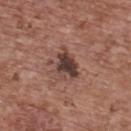This lesion was catalogued during total-body skin photography and was not selected for biopsy. The recorded lesion diameter is about 4 mm. A close-up tile cropped from a whole-body skin photograph, about 15 mm across. A female subject, aged 63 to 67. Captured under white-light illumination. The lesion is located on the upper back. Automated tile analysis of the lesion measured a lesion area of about 9 mm² and an outline eccentricity of about 0.55 (0 = round, 1 = elongated). The analysis additionally found border irregularity of about 5 on a 0–10 scale, a within-lesion color-variation index near 6/10, and radial color variation of about 2. It also reported a nevus-likeness score of about 35/100.